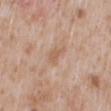This lesion was catalogued during total-body skin photography and was not selected for biopsy. A male subject aged around 50. Automated image analysis of the tile measured a lesion–skin lightness drop of about 7 and a normalized border contrast of about 5.5. It also reported an automated nevus-likeness rating near 0 out of 100 and lesion-presence confidence of about 100/100. This image is a 15 mm lesion crop taken from a total-body photograph. The tile uses white-light illumination. The recorded lesion diameter is about 3 mm. The lesion is on the mid back.